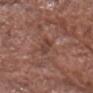Assessment:
The lesion was tiled from a total-body skin photograph and was not biopsied.
Clinical summary:
The lesion is on the head or neck. This image is a 15 mm lesion crop taken from a total-body photograph. About 3 mm across. Captured under white-light illumination. A male subject about 75 years old.